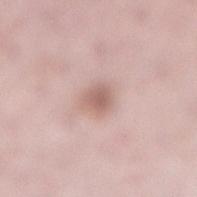| field | value |
|---|---|
| notes | catalogued during a skin exam; not biopsied |
| patient | female, aged 48 to 52 |
| image source | total-body-photography crop, ~15 mm field of view |
| illumination | white-light |
| site | the right lower leg |
| TBP lesion metrics | a footprint of about 4.5 mm², an eccentricity of roughly 0.5, and a shape-asymmetry score of about 0.25 (0 = symmetric); an average lesion color of about L≈61 a*≈19 b*≈23 (CIELAB) and a lesion-to-skin contrast of about 7 (normalized; higher = more distinct); a border-irregularity index near 2/10, a color-variation rating of about 2/10, and radial color variation of about 0.5 |